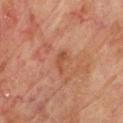Recorded during total-body skin imaging; not selected for excision or biopsy. On the upper back. The tile uses cross-polarized illumination. The subject is a male aged 73–77. A close-up tile cropped from a whole-body skin photograph, about 15 mm across.